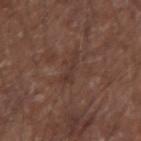Q: Was a biopsy performed?
A: no biopsy performed (imaged during a skin exam)